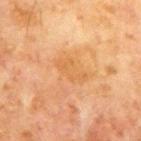<tbp_lesion>
  <biopsy_status>not biopsied; imaged during a skin examination</biopsy_status>
  <lesion_size>
    <long_diameter_mm_approx>4.0</long_diameter_mm_approx>
  </lesion_size>
  <patient>
    <sex>male</sex>
    <age_approx>65</age_approx>
  </patient>
  <image>
    <source>total-body photography crop</source>
    <field_of_view_mm>15</field_of_view_mm>
  </image>
  <site>chest</site>
  <lighting>cross-polarized</lighting>
</tbp_lesion>